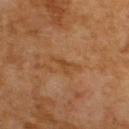<tbp_lesion>
  <biopsy_status>not biopsied; imaged during a skin examination</biopsy_status>
  <site>upper back</site>
  <patient>
    <sex>male</sex>
    <age_approx>65</age_approx>
  </patient>
  <image>
    <source>total-body photography crop</source>
    <field_of_view_mm>15</field_of_view_mm>
  </image>
</tbp_lesion>